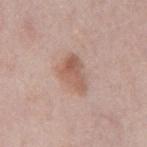workup — imaged on a skin check; not biopsied | image-analysis metrics — an area of roughly 8.5 mm², an outline eccentricity of about 0.75 (0 = round, 1 = elongated), and a shape-asymmetry score of about 0.35 (0 = symmetric); an automated nevus-likeness rating near 50 out of 100 | location — the mid back | illumination — white-light | image source — ~15 mm crop, total-body skin-cancer survey | patient — male, aged 73 to 77.